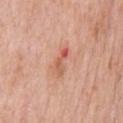The recorded lesion diameter is about 3.5 mm.
On the chest.
A male patient, approximately 60 years of age.
Cropped from a whole-body photographic skin survey; the tile spans about 15 mm.
Imaged with white-light lighting.